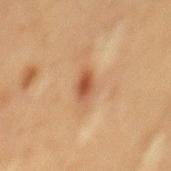workup=imaged on a skin check; not biopsied.